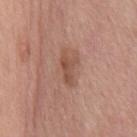Q: Was a biopsy performed?
A: total-body-photography surveillance lesion; no biopsy
Q: Who is the patient?
A: male, in their mid-50s
Q: Illumination type?
A: white-light
Q: Lesion location?
A: the chest
Q: Automated lesion metrics?
A: a footprint of about 4.5 mm², an eccentricity of roughly 0.9, and a shape-asymmetry score of about 0.45 (0 = symmetric); a lesion color around L≈50 a*≈21 b*≈28 in CIELAB, roughly 9 lightness units darker than nearby skin, and a lesion-to-skin contrast of about 7 (normalized; higher = more distinct); a border-irregularity index near 5/10, a color-variation rating of about 2/10, and peripheral color asymmetry of about 0.5
Q: What is the imaging modality?
A: ~15 mm crop, total-body skin-cancer survey